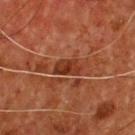Q: Was a biopsy performed?
A: catalogued during a skin exam; not biopsied
Q: Who is the patient?
A: male, aged 53–57
Q: What lighting was used for the tile?
A: cross-polarized
Q: What is the imaging modality?
A: ~15 mm tile from a whole-body skin photo
Q: What is the anatomic site?
A: the chest